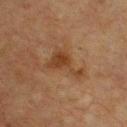Context:
Cropped from a whole-body photographic skin survey; the tile spans about 15 mm. The lesion is on the chest. A male patient aged around 75.